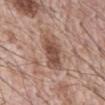notes — imaged on a skin check; not biopsied
diameter — ~4.5 mm (longest diameter)
subject — male, about 70 years old
tile lighting — white-light
site — the abdomen
imaging modality — total-body-photography crop, ~15 mm field of view